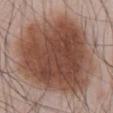* location — the abdomen
* subject — male, approximately 55 years of age
* lighting — white-light
* image-analysis metrics — a mean CIELAB color near L≈47 a*≈20 b*≈26, a lesion–skin lightness drop of about 14, and a normalized border contrast of about 10.5
* acquisition — ~15 mm crop, total-body skin-cancer survey
* lesion diameter — ~12.5 mm (longest diameter)